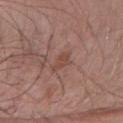Case summary:
– notes · no biopsy performed (imaged during a skin exam)
– subject · male, aged 53–57
– image · ~15 mm tile from a whole-body skin photo
– body site · the right forearm